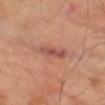No biopsy was performed on this lesion — it was imaged during a full skin examination and was not determined to be concerning.
A lesion tile, about 15 mm wide, cut from a 3D total-body photograph.
A male subject in their 70s.
The lesion is located on the leg.
Measured at roughly 4.5 mm in maximum diameter.
This is a cross-polarized tile.